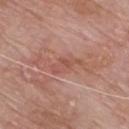* notes — total-body-photography surveillance lesion; no biopsy
* anatomic site — the front of the torso
* patient — male, roughly 60 years of age
* tile lighting — white-light
* imaging modality — total-body-photography crop, ~15 mm field of view